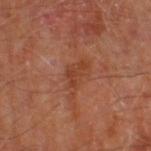Recorded during total-body skin imaging; not selected for excision or biopsy.
A male subject, aged approximately 70.
This image is a 15 mm lesion crop taken from a total-body photograph.
Longest diameter approximately 4.5 mm.
This is a cross-polarized tile.
The lesion-visualizer software estimated an area of roughly 6 mm² and two-axis asymmetry of about 0.5. The analysis additionally found an average lesion color of about L≈41 a*≈25 b*≈32 (CIELAB), a lesion–skin lightness drop of about 7, and a lesion-to-skin contrast of about 6 (normalized; higher = more distinct). And it measured a border-irregularity index near 6.5/10 and peripheral color asymmetry of about 0.5. It also reported an automated nevus-likeness rating near 0 out of 100 and a detector confidence of about 100 out of 100 that the crop contains a lesion.
From the right thigh.